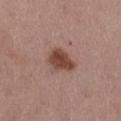• notes · total-body-photography surveillance lesion; no biopsy
• imaging modality · total-body-photography crop, ~15 mm field of view
• patient · female, roughly 55 years of age
• tile lighting · white-light illumination
• anatomic site · the left thigh